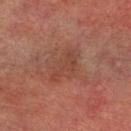follow-up = no biopsy performed (imaged during a skin exam)
anatomic site = the left lower leg
image-analysis metrics = a lesion area of about 8.5 mm²; a classifier nevus-likeness of about 0/100 and a detector confidence of about 100 out of 100 that the crop contains a lesion
tile lighting = cross-polarized illumination
lesion size = about 4.5 mm
image source = ~15 mm tile from a whole-body skin photo
subject = male, about 75 years old